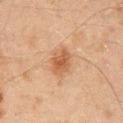Part of a total-body skin-imaging series; this lesion was reviewed on a skin check and was not flagged for biopsy. Cropped from a total-body skin-imaging series; the visible field is about 15 mm. From the mid back. A male subject, in their mid- to late 40s.